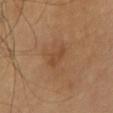Case summary:
* biopsy status · no biopsy performed (imaged during a skin exam)
* acquisition · ~15 mm crop, total-body skin-cancer survey
* location · the chest
* lighting · cross-polarized illumination
* lesion diameter · about 3.5 mm